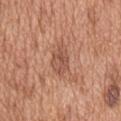Assessment:
Captured during whole-body skin photography for melanoma surveillance; the lesion was not biopsied.
Image and clinical context:
A male patient aged approximately 65. The lesion-visualizer software estimated a footprint of about 7 mm², an outline eccentricity of about 0.85 (0 = round, 1 = elongated), and a shape-asymmetry score of about 0.2 (0 = symmetric). On the mid back. A 15 mm crop from a total-body photograph taken for skin-cancer surveillance. Captured under white-light illumination.